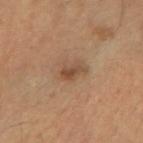notes: imaged on a skin check; not biopsied | site: the right forearm | lesion diameter: ~2.5 mm (longest diameter) | image source: total-body-photography crop, ~15 mm field of view | lighting: cross-polarized | patient: male, aged 53 to 57.